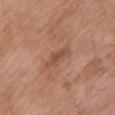The lesion was photographed on a routine skin check and not biopsied; there is no pathology result. A female patient about 70 years old. Located on the upper back. Cropped from a whole-body photographic skin survey; the tile spans about 15 mm.